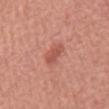Q: Is there a histopathology result?
A: catalogued during a skin exam; not biopsied
Q: Illumination type?
A: white-light illumination
Q: How was this image acquired?
A: ~15 mm crop, total-body skin-cancer survey
Q: Who is the patient?
A: male, aged around 65
Q: What is the lesion's diameter?
A: ≈3.5 mm
Q: Where on the body is the lesion?
A: the abdomen